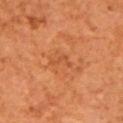No biopsy was performed on this lesion — it was imaged during a full skin examination and was not determined to be concerning. The subject is about 65 years old. A 15 mm close-up extracted from a 3D total-body photography capture. Located on the left upper arm.